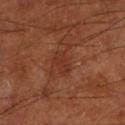| feature | finding |
|---|---|
| notes | catalogued during a skin exam; not biopsied |
| tile lighting | cross-polarized |
| location | the left lower leg |
| patient | male, in their mid- to late 60s |
| image source | total-body-photography crop, ~15 mm field of view |
| lesion size | about 3 mm |